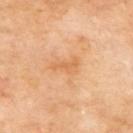No biopsy was performed on this lesion — it was imaged during a full skin examination and was not determined to be concerning. A roughly 15 mm field-of-view crop from a total-body skin photograph. Captured under cross-polarized illumination. Located on the upper back. Measured at roughly 3 mm in maximum diameter. A male subject, approximately 70 years of age.